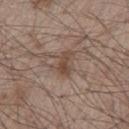Impression: Captured during whole-body skin photography for melanoma surveillance; the lesion was not biopsied. Clinical summary: A region of skin cropped from a whole-body photographic capture, roughly 15 mm wide. The patient is a male in their 80s. The lesion's longest dimension is about 3.5 mm. From the chest. Captured under white-light illumination.